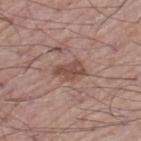<case>
<biopsy_status>not biopsied; imaged during a skin examination</biopsy_status>
</case>